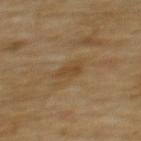The lesion was photographed on a routine skin check and not biopsied; there is no pathology result.
The lesion is located on the back.
The subject is a male roughly 70 years of age.
A lesion tile, about 15 mm wide, cut from a 3D total-body photograph.